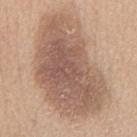Q: Who is the patient?
A: male, aged around 60
Q: Where on the body is the lesion?
A: the mid back
Q: How large is the lesion?
A: about 12 mm
Q: What kind of image is this?
A: total-body-photography crop, ~15 mm field of view
Q: How was the tile lit?
A: white-light